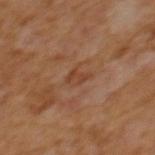• notes · catalogued during a skin exam; not biopsied
• patient · male, in their mid- to late 60s
• tile lighting · cross-polarized
• acquisition · ~15 mm crop, total-body skin-cancer survey
• image-analysis metrics · a border-irregularity index near 4/10, internal color variation of about 2 on a 0–10 scale, and peripheral color asymmetry of about 0.5; a classifier nevus-likeness of about 0/100 and lesion-presence confidence of about 100/100
• location · the mid back
• size · ~3 mm (longest diameter)